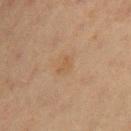Assessment:
Part of a total-body skin-imaging series; this lesion was reviewed on a skin check and was not flagged for biopsy.
Context:
Located on the right thigh. The subject is a female aged around 50. Automated image analysis of the tile measured a lesion color around L≈46 a*≈15 b*≈30 in CIELAB and a normalized border contrast of about 4.5. The software also gave internal color variation of about 2.5 on a 0–10 scale. The analysis additionally found a nevus-likeness score of about 0/100. A lesion tile, about 15 mm wide, cut from a 3D total-body photograph.